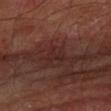<record>
<biopsy_status>not biopsied; imaged during a skin examination</biopsy_status>
<automated_metrics>
  <area_mm2_approx>4.5</area_mm2_approx>
  <eccentricity>0.65</eccentricity>
  <color_variation_0_10>1.5</color_variation_0_10>
  <peripheral_color_asymmetry>0.5</peripheral_color_asymmetry>
  <nevus_likeness_0_100>0</nevus_likeness_0_100>
  <lesion_detection_confidence_0_100>80</lesion_detection_confidence_0_100>
</automated_metrics>
<patient>
  <sex>male</sex>
  <age_approx>70</age_approx>
</patient>
<lesion_size>
  <long_diameter_mm_approx>3.0</long_diameter_mm_approx>
</lesion_size>
<image>
  <source>total-body photography crop</source>
  <field_of_view_mm>15</field_of_view_mm>
</image>
<lighting>cross-polarized</lighting>
<site>left forearm</site>
</record>